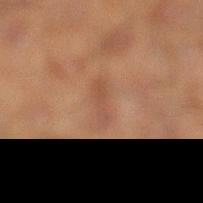workup = total-body-photography surveillance lesion; no biopsy
subject = male, aged approximately 50
imaging modality = ~15 mm tile from a whole-body skin photo
automated metrics = a mean CIELAB color near L≈38 a*≈17 b*≈25, a lesion–skin lightness drop of about 5, and a normalized border contrast of about 4.5; a border-irregularity index near 5/10, a within-lesion color-variation index near 1/10, and radial color variation of about 0; an automated nevus-likeness rating near 0 out of 100 and a detector confidence of about 100 out of 100 that the crop contains a lesion
site = the left lower leg
size = ≈3.5 mm
illumination = cross-polarized